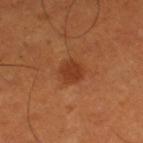Notes:
– tile lighting · cross-polarized
– site · the left thigh
– subject · male, about 50 years old
– acquisition · 15 mm crop, total-body photography
– lesion diameter · ≈2.5 mm